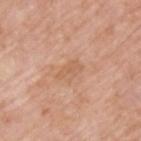notes — imaged on a skin check; not biopsied
lighting — white-light
image-analysis metrics — a shape eccentricity near 0.9; a lesion color around L≈61 a*≈21 b*≈34 in CIELAB, about 6 CIELAB-L* units darker than the surrounding skin, and a normalized lesion–skin contrast near 5; an automated nevus-likeness rating near 0 out of 100
acquisition — total-body-photography crop, ~15 mm field of view
subject — male, aged 63 to 67
site — the back